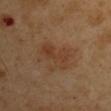{"biopsy_status": "not biopsied; imaged during a skin examination", "image": {"source": "total-body photography crop", "field_of_view_mm": 15}, "patient": {"sex": "male", "age_approx": 50}, "site": "right upper arm", "automated_metrics": {"cielab_L": 38, "cielab_a": 19, "cielab_b": 31, "vs_skin_darker_L": 6.0, "vs_skin_contrast_norm": 5.5}}